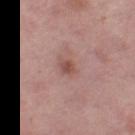Impression: No biopsy was performed on this lesion — it was imaged during a full skin examination and was not determined to be concerning. Background: On the left thigh. Captured under white-light illumination. Cropped from a whole-body photographic skin survey; the tile spans about 15 mm. A female subject, aged around 50. Approximately 2.5 mm at its widest.